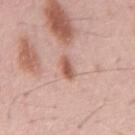notes — imaged on a skin check; not biopsied
automated metrics — an area of roughly 4 mm², an eccentricity of roughly 0.85, and two-axis asymmetry of about 0.25; a lesion color around L≈58 a*≈23 b*≈28 in CIELAB, a lesion–skin lightness drop of about 12, and a lesion-to-skin contrast of about 8 (normalized; higher = more distinct)
patient — male, in their mid-50s
lighting — white-light illumination
acquisition — 15 mm crop, total-body photography